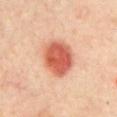No biopsy was performed on this lesion — it was imaged during a full skin examination and was not determined to be concerning. Longest diameter approximately 4.5 mm. This is a cross-polarized tile. From the chest. Cropped from a total-body skin-imaging series; the visible field is about 15 mm. An algorithmic analysis of the crop reported border irregularity of about 1 on a 0–10 scale, a color-variation rating of about 5/10, and a peripheral color-asymmetry measure near 1.5. And it measured a classifier nevus-likeness of about 100/100 and lesion-presence confidence of about 100/100. A female patient, in their mid-40s.